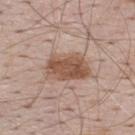Captured during whole-body skin photography for melanoma surveillance; the lesion was not biopsied.
Cropped from a total-body skin-imaging series; the visible field is about 15 mm.
A male subject in their 70s.
The lesion-visualizer software estimated a border-irregularity rating of about 2.5/10, a within-lesion color-variation index near 4.5/10, and a peripheral color-asymmetry measure near 1.5. It also reported a nevus-likeness score of about 100/100 and a detector confidence of about 100 out of 100 that the crop contains a lesion.
The recorded lesion diameter is about 5 mm.
The tile uses white-light illumination.
The lesion is located on the upper back.